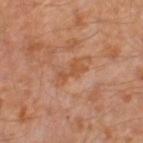notes: total-body-photography surveillance lesion; no biopsy | patient: male, approximately 30 years of age | image source: ~15 mm tile from a whole-body skin photo | site: the left thigh.